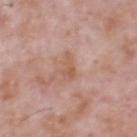Assessment: The lesion was tiled from a total-body skin photograph and was not biopsied. Clinical summary: From the head or neck. A male subject, approximately 70 years of age. The lesion's longest dimension is about 3.5 mm. Captured under white-light illumination. The total-body-photography lesion software estimated a lesion color around L≈57 a*≈21 b*≈30 in CIELAB, a lesion–skin lightness drop of about 7, and a normalized lesion–skin contrast near 6.5. A lesion tile, about 15 mm wide, cut from a 3D total-body photograph.